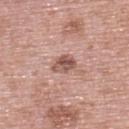Q: Was a biopsy performed?
A: imaged on a skin check; not biopsied
Q: What is the imaging modality?
A: ~15 mm crop, total-body skin-cancer survey
Q: Patient demographics?
A: female, aged around 60
Q: Illumination type?
A: white-light illumination
Q: Where on the body is the lesion?
A: the upper back
Q: Automated lesion metrics?
A: a mean CIELAB color near L≈54 a*≈21 b*≈25 and a normalized border contrast of about 8.5; an automated nevus-likeness rating near 30 out of 100 and a detector confidence of about 100 out of 100 that the crop contains a lesion